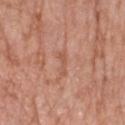Case summary:
* notes · imaged on a skin check; not biopsied
* patient · female, approximately 75 years of age
* image · ~15 mm crop, total-body skin-cancer survey
* size · about 3 mm
* location · the arm
* automated lesion analysis · a footprint of about 3.5 mm² and two-axis asymmetry of about 0.4; a mean CIELAB color near L≈56 a*≈23 b*≈31; a border-irregularity rating of about 4.5/10, a color-variation rating of about 0.5/10, and peripheral color asymmetry of about 0
* illumination · white-light